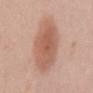Case summary:
• automated metrics — an area of roughly 25 mm², an outline eccentricity of about 0.85 (0 = round, 1 = elongated), and two-axis asymmetry of about 0.1; border irregularity of about 1.5 on a 0–10 scale, a within-lesion color-variation index near 3.5/10, and peripheral color asymmetry of about 1; a classifier nevus-likeness of about 95/100 and a lesion-detection confidence of about 100/100
• image source — ~15 mm tile from a whole-body skin photo
• site — the mid back
• patient — female, aged 58 to 62
• illumination — white-light illumination